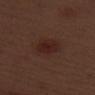image source: 15 mm crop, total-body photography; patient: male, in their 70s; tile lighting: white-light; location: the arm; lesion diameter: ~4 mm (longest diameter).